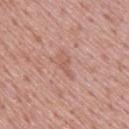{"biopsy_status": "not biopsied; imaged during a skin examination", "patient": {"sex": "male", "age_approx": 75}, "lesion_size": {"long_diameter_mm_approx": 3.0}, "site": "upper back", "lighting": "white-light", "image": {"source": "total-body photography crop", "field_of_view_mm": 15}}